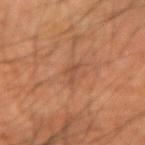Case summary:
• biopsy status · no biopsy performed (imaged during a skin exam)
• imaging modality · ~15 mm tile from a whole-body skin photo
• patient · male, in their 60s
• location · the arm
• lesion diameter · ≈2.5 mm
• TBP lesion metrics · a lesion color around L≈44 a*≈21 b*≈30 in CIELAB, a lesion–skin lightness drop of about 6, and a normalized border contrast of about 5; a classifier nevus-likeness of about 0/100 and lesion-presence confidence of about 80/100